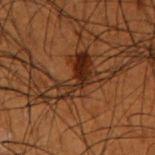Part of a total-body skin-imaging series; this lesion was reviewed on a skin check and was not flagged for biopsy. An algorithmic analysis of the crop reported a lesion color around L≈23 a*≈17 b*≈24 in CIELAB and a normalized border contrast of about 9. It also reported border irregularity of about 9 on a 0–10 scale, a color-variation rating of about 8.5/10, and radial color variation of about 3. The analysis additionally found an automated nevus-likeness rating near 100 out of 100 and a detector confidence of about 65 out of 100 that the crop contains a lesion. The recorded lesion diameter is about 7.5 mm. The patient is a male aged approximately 50. A close-up tile cropped from a whole-body skin photograph, about 15 mm across. On the left forearm.